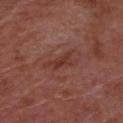| feature | finding |
|---|---|
| biopsy status | total-body-photography surveillance lesion; no biopsy |
| illumination | white-light illumination |
| subject | male, in their mid-60s |
| image | ~15 mm tile from a whole-body skin photo |
| image-analysis metrics | an area of roughly 4.5 mm², a shape eccentricity near 0.85, and two-axis asymmetry of about 0.5; a lesion color around L≈36 a*≈25 b*≈26 in CIELAB and a lesion-to-skin contrast of about 6.5 (normalized; higher = more distinct); a classifier nevus-likeness of about 0/100 and lesion-presence confidence of about 100/100 |
| location | the front of the torso |